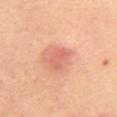No biopsy was performed on this lesion — it was imaged during a full skin examination and was not determined to be concerning. Approximately 3 mm at its widest. The lesion is on the lower back. The patient is a male in their mid- to late 60s. Cropped from a total-body skin-imaging series; the visible field is about 15 mm. Captured under cross-polarized illumination.